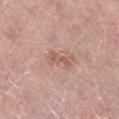Acquisition and patient details: Imaged with white-light lighting. An algorithmic analysis of the crop reported a footprint of about 3.5 mm², a shape eccentricity near 0.9, and a shape-asymmetry score of about 0.5 (0 = symmetric). The analysis additionally found an average lesion color of about L≈57 a*≈22 b*≈27 (CIELAB), about 8 CIELAB-L* units darker than the surrounding skin, and a normalized lesion–skin contrast near 6. And it measured a border-irregularity index near 5.5/10, internal color variation of about 0.5 on a 0–10 scale, and peripheral color asymmetry of about 0. The lesion is located on the left lower leg. A 15 mm crop from a total-body photograph taken for skin-cancer surveillance. The lesion's longest dimension is about 3 mm. A female patient, in their mid-60s.